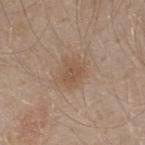Assessment: The lesion was photographed on a routine skin check and not biopsied; there is no pathology result. Clinical summary: A region of skin cropped from a whole-body photographic capture, roughly 15 mm wide. Located on the upper back. Captured under white-light illumination. Approximately 3 mm at its widest. A male subject, roughly 30 years of age.